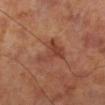follow-up = imaged on a skin check; not biopsied
automated metrics = a symmetry-axis asymmetry near 0.55; a normalized border contrast of about 6.5; border irregularity of about 6 on a 0–10 scale and a color-variation rating of about 3/10; a lesion-detection confidence of about 100/100
tile lighting = cross-polarized illumination
subject = male, in their 70s
location = the left lower leg
size = about 4.5 mm
acquisition = ~15 mm crop, total-body skin-cancer survey